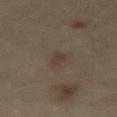Q: Is there a histopathology result?
A: imaged on a skin check; not biopsied